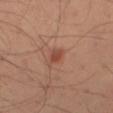notes: total-body-photography surveillance lesion; no biopsy
TBP lesion metrics: a lesion area of about 3 mm² and two-axis asymmetry of about 0.3; internal color variation of about 2.5 on a 0–10 scale and radial color variation of about 1; a classifier nevus-likeness of about 90/100 and a lesion-detection confidence of about 100/100
imaging modality: ~15 mm crop, total-body skin-cancer survey
lesion diameter: ~2.5 mm (longest diameter)
site: the right thigh
patient: male, approximately 55 years of age